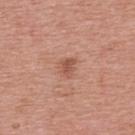Captured during whole-body skin photography for melanoma surveillance; the lesion was not biopsied. A roughly 15 mm field-of-view crop from a total-body skin photograph. Approximately 2.5 mm at its widest. The tile uses white-light illumination. The lesion is on the upper back. A female subject, approximately 70 years of age.